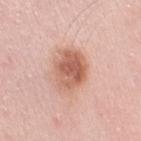The lesion-visualizer software estimated a lesion color around L≈61 a*≈24 b*≈30 in CIELAB, roughly 13 lightness units darker than nearby skin, and a normalized lesion–skin contrast near 8.5. The analysis additionally found a border-irregularity rating of about 2.5/10, internal color variation of about 5.5 on a 0–10 scale, and radial color variation of about 2. The analysis additionally found an automated nevus-likeness rating near 85 out of 100 and a lesion-detection confidence of about 100/100.
The tile uses white-light illumination.
A female subject, roughly 40 years of age.
On the right upper arm.
A roughly 15 mm field-of-view crop from a total-body skin photograph.
Measured at roughly 5 mm in maximum diameter.